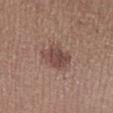{
  "biopsy_status": "not biopsied; imaged during a skin examination",
  "image": {
    "source": "total-body photography crop",
    "field_of_view_mm": 15
  },
  "site": "left lower leg",
  "lighting": "white-light",
  "patient": {
    "sex": "male",
    "age_approx": 65
  },
  "lesion_size": {
    "long_diameter_mm_approx": 3.5
  }
}